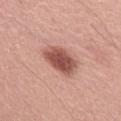Captured during whole-body skin photography for melanoma surveillance; the lesion was not biopsied. Cropped from a whole-body photographic skin survey; the tile spans about 15 mm. The patient is a female aged around 60. The lesion is on the abdomen. About 4.5 mm across.